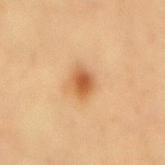Clinical impression: Recorded during total-body skin imaging; not selected for excision or biopsy. Background: The lesion is on the mid back. A female subject in their mid- to late 40s. A roughly 15 mm field-of-view crop from a total-body skin photograph. The lesion-visualizer software estimated a lesion area of about 6 mm² and two-axis asymmetry of about 0.25. And it measured an average lesion color of about L≈59 a*≈24 b*≈41 (CIELAB). And it measured a border-irregularity index near 2/10 and peripheral color asymmetry of about 1. The analysis additionally found a detector confidence of about 100 out of 100 that the crop contains a lesion. Imaged with cross-polarized lighting.